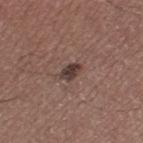workup — imaged on a skin check; not biopsied | diameter — ≈2.5 mm | acquisition — 15 mm crop, total-body photography | subject — male, about 45 years old | illumination — white-light | TBP lesion metrics — a lesion area of about 3.5 mm² and a shape-asymmetry score of about 0.25 (0 = symmetric); an average lesion color of about L≈37 a*≈16 b*≈19 (CIELAB), about 12 CIELAB-L* units darker than the surrounding skin, and a normalized lesion–skin contrast near 10.5; border irregularity of about 2 on a 0–10 scale and peripheral color asymmetry of about 1; a classifier nevus-likeness of about 45/100 | location — the left thigh.